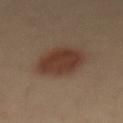Assessment:
The lesion was tiled from a total-body skin photograph and was not biopsied.
Acquisition and patient details:
Located on the abdomen. The patient is a male about 40 years old. Cropped from a whole-body photographic skin survey; the tile spans about 15 mm.